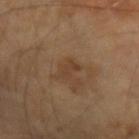The lesion was photographed on a routine skin check and not biopsied; there is no pathology result.
The lesion is located on the left forearm.
A male patient aged 63 to 67.
Automated tile analysis of the lesion measured a symmetry-axis asymmetry near 0.35. It also reported a lesion-detection confidence of about 100/100.
A 15 mm crop from a total-body photograph taken for skin-cancer surveillance.